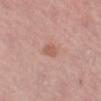| field | value |
|---|---|
| subject | female, aged approximately 50 |
| site | the left thigh |
| automated lesion analysis | a mean CIELAB color near L≈58 a*≈23 b*≈28, roughly 8 lightness units darker than nearby skin, and a lesion-to-skin contrast of about 6.5 (normalized; higher = more distinct); border irregularity of about 2 on a 0–10 scale |
| imaging modality | total-body-photography crop, ~15 mm field of view |